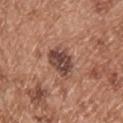The patient is a male about 55 years old.
Longest diameter approximately 3.5 mm.
The lesion-visualizer software estimated an area of roughly 9 mm², a shape eccentricity near 0.5, and a symmetry-axis asymmetry near 0.25. It also reported a mean CIELAB color near L≈45 a*≈21 b*≈25, about 13 CIELAB-L* units darker than the surrounding skin, and a normalized lesion–skin contrast near 10. It also reported a border-irregularity index near 2.5/10 and peripheral color asymmetry of about 2. The analysis additionally found a classifier nevus-likeness of about 5/100 and a detector confidence of about 100 out of 100 that the crop contains a lesion.
The tile uses white-light illumination.
The lesion is on the upper back.
A close-up tile cropped from a whole-body skin photograph, about 15 mm across.